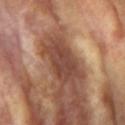workup: total-body-photography surveillance lesion; no biopsy
automated lesion analysis: a lesion area of about 12 mm² and an outline eccentricity of about 0.8 (0 = round, 1 = elongated); a mean CIELAB color near L≈43 a*≈22 b*≈27, about 11 CIELAB-L* units darker than the surrounding skin, and a normalized border contrast of about 9; an automated nevus-likeness rating near 0 out of 100 and a detector confidence of about 85 out of 100 that the crop contains a lesion
body site: the left upper arm
lesion diameter: about 5 mm
image: 15 mm crop, total-body photography
subject: female, roughly 75 years of age